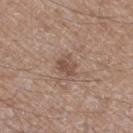biopsy status: no biopsy performed (imaged during a skin exam) | diameter: ~2.5 mm (longest diameter) | patient: male, in their mid- to late 60s | site: the right thigh | imaging modality: total-body-photography crop, ~15 mm field of view.